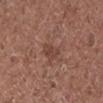Notes:
* follow-up: imaged on a skin check; not biopsied
* tile lighting: white-light illumination
* subject: female, in their 50s
* body site: the right lower leg
* acquisition: 15 mm crop, total-body photography
* size: ~3 mm (longest diameter)